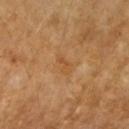Impression: The lesion was tiled from a total-body skin photograph and was not biopsied. Clinical summary: The total-body-photography lesion software estimated an area of roughly 3 mm². And it measured a mean CIELAB color near L≈53 a*≈22 b*≈41, roughly 6 lightness units darker than nearby skin, and a normalized lesion–skin contrast near 5. And it measured a border-irregularity index near 5/10 and radial color variation of about 0. Captured under cross-polarized illumination. The lesion's longest dimension is about 2.5 mm. Located on the right forearm. Cropped from a whole-body photographic skin survey; the tile spans about 15 mm. A female subject, in their 70s.